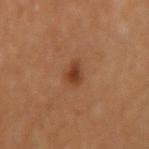notes: imaged on a skin check; not biopsied | body site: the arm | lesion size: ≈2 mm | imaging modality: 15 mm crop, total-body photography | patient: female, in their mid- to late 50s | tile lighting: cross-polarized illumination | automated lesion analysis: an average lesion color of about L≈37 a*≈22 b*≈31 (CIELAB) and roughly 9 lightness units darker than nearby skin; a color-variation rating of about 2.5/10 and a peripheral color-asymmetry measure near 1; a classifier nevus-likeness of about 90/100 and lesion-presence confidence of about 100/100.